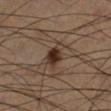Background:
About 3.5 mm across. A 15 mm crop from a total-body photograph taken for skin-cancer surveillance. From the right lower leg. Automated tile analysis of the lesion measured an area of roughly 6 mm², a shape eccentricity near 0.75, and a symmetry-axis asymmetry near 0.3. The software also gave a border-irregularity rating of about 3/10, internal color variation of about 4 on a 0–10 scale, and radial color variation of about 1.5. And it measured a nevus-likeness score of about 100/100 and lesion-presence confidence of about 100/100. A male patient roughly 65 years of age.